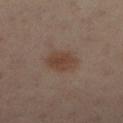Findings:
* follow-up · imaged on a skin check; not biopsied
* site · the left leg
* size · ~3.5 mm (longest diameter)
* image · total-body-photography crop, ~15 mm field of view
* illumination · cross-polarized illumination
* TBP lesion metrics · roughly 8 lightness units darker than nearby skin and a lesion-to-skin contrast of about 7.5 (normalized; higher = more distinct); border irregularity of about 2 on a 0–10 scale and a within-lesion color-variation index near 2.5/10
* subject · female, roughly 40 years of age